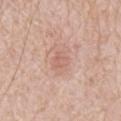Background:
A male subject, aged around 80. A region of skin cropped from a whole-body photographic capture, roughly 15 mm wide. The lesion is on the mid back.